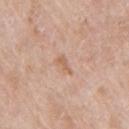biopsy_status: not biopsied; imaged during a skin examination
lighting: white-light
automated_metrics:
  eccentricity: 0.85
  vs_skin_darker_L: 7.0
  vs_skin_contrast_norm: 6.0
image:
  source: total-body photography crop
  field_of_view_mm: 15
site: right upper arm
patient:
  sex: male
  age_approx: 65